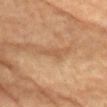Assessment: Captured during whole-body skin photography for melanoma surveillance; the lesion was not biopsied. Context: A close-up tile cropped from a whole-body skin photograph, about 15 mm across. A female patient, aged 78–82. The lesion is on the chest.